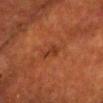Recorded during total-body skin imaging; not selected for excision or biopsy. A 15 mm close-up extracted from a 3D total-body photography capture. The lesion is on the head or neck. The tile uses cross-polarized illumination. Approximately 2.5 mm at its widest. A male subject, in their mid- to late 60s.